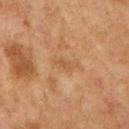Imaged during a routine full-body skin examination; the lesion was not biopsied and no histopathology is available.
A female subject aged approximately 60.
Located on the right upper arm.
Longest diameter approximately 2.5 mm.
Cropped from a total-body skin-imaging series; the visible field is about 15 mm.
Captured under cross-polarized illumination.